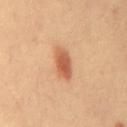Context:
Approximately 4 mm at its widest. An algorithmic analysis of the crop reported a footprint of about 7 mm², an outline eccentricity of about 0.8 (0 = round, 1 = elongated), and two-axis asymmetry of about 0.2. The software also gave a mean CIELAB color near L≈58 a*≈26 b*≈36, a lesion–skin lightness drop of about 12, and a lesion-to-skin contrast of about 8 (normalized; higher = more distinct). And it measured a border-irregularity index near 2/10, a within-lesion color-variation index near 4/10, and a peripheral color-asymmetry measure near 1.5. This is a cross-polarized tile. A female patient aged 48–52. A 15 mm close-up extracted from a 3D total-body photography capture.